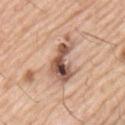No biopsy was performed on this lesion — it was imaged during a full skin examination and was not determined to be concerning. The patient is a male in their mid-50s. This image is a 15 mm lesion crop taken from a total-body photograph. On the left upper arm. Measured at roughly 5.5 mm in maximum diameter.